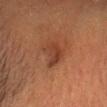follow-up: no biopsy performed (imaged during a skin exam)
body site: the head or neck
patient: male, aged 58 to 62
image source: ~15 mm tile from a whole-body skin photo